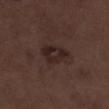Imaged during a routine full-body skin examination; the lesion was not biopsied and no histopathology is available.
Longest diameter approximately 3.5 mm.
A region of skin cropped from a whole-body photographic capture, roughly 15 mm wide.
On the left lower leg.
This is a white-light tile.
A male subject in their 70s.